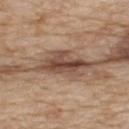Case summary:
• biopsy status — imaged on a skin check; not biopsied
• subject — male, approximately 80 years of age
• tile lighting — white-light illumination
• lesion size — ≈6.5 mm
• location — the upper back
• acquisition — 15 mm crop, total-body photography